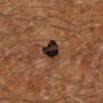{"biopsy_status": "not biopsied; imaged during a skin examination", "image": {"source": "total-body photography crop", "field_of_view_mm": 15}, "lighting": "cross-polarized", "site": "left lower leg", "lesion_size": {"long_diameter_mm_approx": 3.0}, "patient": {"sex": "male", "age_approx": 60}}